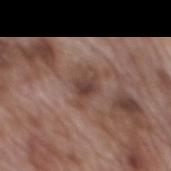Impression: Captured during whole-body skin photography for melanoma surveillance; the lesion was not biopsied. Acquisition and patient details: Longest diameter approximately 3.5 mm. The lesion is on the mid back. A male patient approximately 70 years of age. This image is a 15 mm lesion crop taken from a total-body photograph. This is a white-light tile. The lesion-visualizer software estimated a lesion–skin lightness drop of about 9. It also reported a nevus-likeness score of about 0/100 and lesion-presence confidence of about 95/100.